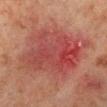Imaged during a routine full-body skin examination; the lesion was not biopsied and no histopathology is available.
Cropped from a total-body skin-imaging series; the visible field is about 15 mm.
The subject is a male aged 63–67.
Measured at roughly 8.5 mm in maximum diameter.
The lesion is located on the right lower leg.